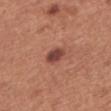Imaged during a routine full-body skin examination; the lesion was not biopsied and no histopathology is available.
On the chest.
The patient is a female aged approximately 50.
A region of skin cropped from a whole-body photographic capture, roughly 15 mm wide.